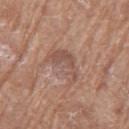The lesion was tiled from a total-body skin photograph and was not biopsied. On the left thigh. Cropped from a whole-body photographic skin survey; the tile spans about 15 mm. A female subject, in their mid-70s.